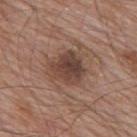Impression: The lesion was tiled from a total-body skin photograph and was not biopsied. Image and clinical context: A male subject, about 80 years old. The lesion is located on the mid back. A close-up tile cropped from a whole-body skin photograph, about 15 mm across.